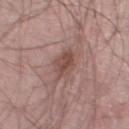Impression: Recorded during total-body skin imaging; not selected for excision or biopsy.